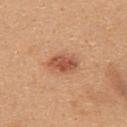This lesion was catalogued during total-body skin photography and was not selected for biopsy.
Approximately 4 mm at its widest.
A female patient aged approximately 25.
This image is a 15 mm lesion crop taken from a total-body photograph.
Located on the back.